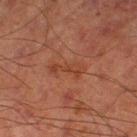biopsy_status: not biopsied; imaged during a skin examination
image:
  source: total-body photography crop
  field_of_view_mm: 15
patient:
  sex: male
  age_approx: 65
lesion_size:
  long_diameter_mm_approx: 4.5
site: left thigh
lighting: cross-polarized